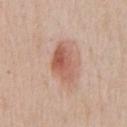{
  "biopsy_status": "not biopsied; imaged during a skin examination",
  "site": "front of the torso",
  "image": {
    "source": "total-body photography crop",
    "field_of_view_mm": 15
  },
  "lesion_size": {
    "long_diameter_mm_approx": 4.5
  },
  "lighting": "white-light",
  "patient": {
    "sex": "male",
    "age_approx": 60
  },
  "automated_metrics": {
    "cielab_L": 58,
    "cielab_a": 24,
    "cielab_b": 29,
    "vs_skin_darker_L": 11.0,
    "vs_skin_contrast_norm": 7.5
  }
}